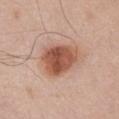<case>
  <biopsy_status>not biopsied; imaged during a skin examination</biopsy_status>
  <image>
    <source>total-body photography crop</source>
    <field_of_view_mm>15</field_of_view_mm>
  </image>
  <site>front of the torso</site>
  <patient>
    <sex>male</sex>
    <age_approx>50</age_approx>
  </patient>
</case>